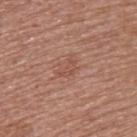Q: Was a biopsy performed?
A: imaged on a skin check; not biopsied
Q: Lesion size?
A: ~2.5 mm (longest diameter)
Q: Patient demographics?
A: male, roughly 55 years of age
Q: Automated lesion metrics?
A: two-axis asymmetry of about 0.5; a lesion color around L≈51 a*≈24 b*≈28 in CIELAB, a lesion–skin lightness drop of about 6, and a normalized border contrast of about 4.5; a nevus-likeness score of about 0/100
Q: What is the imaging modality?
A: total-body-photography crop, ~15 mm field of view
Q: What lighting was used for the tile?
A: white-light illumination
Q: Lesion location?
A: the upper back